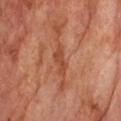Impression: No biopsy was performed on this lesion — it was imaged during a full skin examination and was not determined to be concerning. Context: On the upper back. A male patient, aged 73–77. A lesion tile, about 15 mm wide, cut from a 3D total-body photograph. Captured under cross-polarized illumination. About 3.5 mm across.